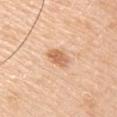Q: Is there a histopathology result?
A: total-body-photography surveillance lesion; no biopsy
Q: How large is the lesion?
A: about 3 mm
Q: Where on the body is the lesion?
A: the arm
Q: Patient demographics?
A: male, aged around 60
Q: What did automated image analysis measure?
A: a border-irregularity index near 2/10; an automated nevus-likeness rating near 80 out of 100 and a detector confidence of about 100 out of 100 that the crop contains a lesion
Q: How was this image acquired?
A: ~15 mm tile from a whole-body skin photo